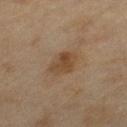{"biopsy_status": "not biopsied; imaged during a skin examination", "patient": {"sex": "female", "age_approx": 60}, "automated_metrics": {"area_mm2_approx": 7.5, "eccentricity": 0.65, "shape_asymmetry": 0.3, "cielab_L": 40, "cielab_a": 15, "cielab_b": 29, "vs_skin_darker_L": 7.0, "nevus_likeness_0_100": 30, "lesion_detection_confidence_0_100": 100}, "lesion_size": {"long_diameter_mm_approx": 3.5}, "site": "left thigh", "image": {"source": "total-body photography crop", "field_of_view_mm": 15}}